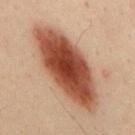Imaged during a routine full-body skin examination; the lesion was not biopsied and no histopathology is available. About 11.5 mm across. Captured under cross-polarized illumination. Automated image analysis of the tile measured an area of roughly 39 mm², a shape eccentricity near 0.9, and two-axis asymmetry of about 0.15. The software also gave a mean CIELAB color near L≈43 a*≈23 b*≈29, roughly 18 lightness units darker than nearby skin, and a normalized lesion–skin contrast near 13. The analysis additionally found border irregularity of about 2.5 on a 0–10 scale, a within-lesion color-variation index near 6.5/10, and peripheral color asymmetry of about 2. The analysis additionally found a classifier nevus-likeness of about 100/100 and lesion-presence confidence of about 100/100. A male subject roughly 50 years of age. On the back. A 15 mm crop from a total-body photograph taken for skin-cancer surveillance.